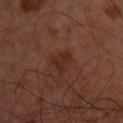Captured during whole-body skin photography for melanoma surveillance; the lesion was not biopsied. Measured at roughly 3 mm in maximum diameter. The tile uses cross-polarized illumination. The lesion-visualizer software estimated a border-irregularity index near 4/10, a color-variation rating of about 1.5/10, and a peripheral color-asymmetry measure near 0.5. A male patient, aged 48 to 52. This image is a 15 mm lesion crop taken from a total-body photograph. Located on the upper back.